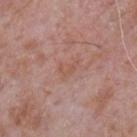Q: Was a biopsy performed?
A: catalogued during a skin exam; not biopsied
Q: What did automated image analysis measure?
A: a footprint of about 3.5 mm², an outline eccentricity of about 0.85 (0 = round, 1 = elongated), and a shape-asymmetry score of about 0.5 (0 = symmetric); a mean CIELAB color near L≈54 a*≈22 b*≈27
Q: What lighting was used for the tile?
A: white-light
Q: Where on the body is the lesion?
A: the upper back
Q: What kind of image is this?
A: 15 mm crop, total-body photography
Q: How large is the lesion?
A: ≈3 mm
Q: Who is the patient?
A: male, aged 63–67